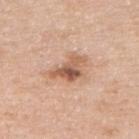| feature | finding |
|---|---|
| notes | total-body-photography surveillance lesion; no biopsy |
| TBP lesion metrics | an automated nevus-likeness rating near 35 out of 100 and a lesion-detection confidence of about 100/100 |
| subject | female, about 45 years old |
| diameter | ~4 mm (longest diameter) |
| illumination | white-light |
| site | the right upper arm |
| image | ~15 mm tile from a whole-body skin photo |